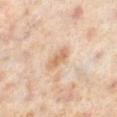The lesion is located on the right lower leg. Automated image analysis of the tile measured an area of roughly 4.5 mm², an eccentricity of roughly 0.85, and two-axis asymmetry of about 0.25. And it measured a classifier nevus-likeness of about 10/100 and a detector confidence of about 100 out of 100 that the crop contains a lesion. A close-up tile cropped from a whole-body skin photograph, about 15 mm across. A female patient, about 50 years old.